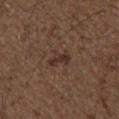biopsy status — catalogued during a skin exam; not biopsied
diameter — about 2.5 mm
anatomic site — the right upper arm
image-analysis metrics — an eccentricity of roughly 0.8 and two-axis asymmetry of about 0.35; a border-irregularity rating of about 4/10 and peripheral color asymmetry of about 0.5
lighting — white-light illumination
acquisition — total-body-photography crop, ~15 mm field of view
subject — male, aged around 50